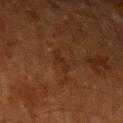Assessment: The lesion was photographed on a routine skin check and not biopsied; there is no pathology result. Clinical summary: A lesion tile, about 15 mm wide, cut from a 3D total-body photograph. The tile uses cross-polarized illumination. Longest diameter approximately 2.5 mm. An algorithmic analysis of the crop reported a lesion area of about 3 mm², a shape eccentricity near 0.8, and two-axis asymmetry of about 0.5. The software also gave a classifier nevus-likeness of about 0/100 and a lesion-detection confidence of about 100/100. A male patient, aged around 60. From the left upper arm.